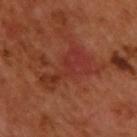Notes:
* automated lesion analysis: a footprint of about 13 mm², an outline eccentricity of about 0.9 (0 = round, 1 = elongated), and two-axis asymmetry of about 0.6; a mean CIELAB color near L≈34 a*≈29 b*≈29 and roughly 6 lightness units darker than nearby skin
* location: the upper back
* illumination: cross-polarized illumination
* subject: male, in their 50s
* image: ~15 mm tile from a whole-body skin photo
* lesion size: ~6.5 mm (longest diameter)